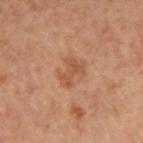biopsy status = catalogued during a skin exam; not biopsied
anatomic site = the right upper arm
tile lighting = cross-polarized illumination
subject = female, roughly 45 years of age
imaging modality = total-body-photography crop, ~15 mm field of view